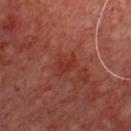Clinical impression:
Imaged during a routine full-body skin examination; the lesion was not biopsied and no histopathology is available.
Clinical summary:
On the chest. A region of skin cropped from a whole-body photographic capture, roughly 15 mm wide. A male patient about 65 years old.